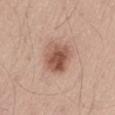biopsy status — no biopsy performed (imaged during a skin exam); image source — total-body-photography crop, ~15 mm field of view; body site — the abdomen; tile lighting — white-light; patient — male, aged 53–57; lesion size — ≈4.5 mm.